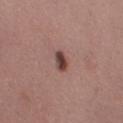Clinical impression: This lesion was catalogued during total-body skin photography and was not selected for biopsy. Clinical summary: The subject is a female in their 40s. Cropped from a whole-body photographic skin survey; the tile spans about 15 mm. From the left thigh.